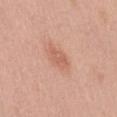The patient is a female roughly 40 years of age.
The lesion is on the lower back.
A 15 mm crop from a total-body photograph taken for skin-cancer surveillance.